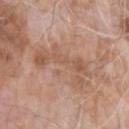Context:
A male subject approximately 75 years of age. Imaged with white-light lighting. Approximately 6.5 mm at its widest. Cropped from a total-body skin-imaging series; the visible field is about 15 mm. Automated tile analysis of the lesion measured a lesion–skin lightness drop of about 8 and a normalized lesion–skin contrast near 5.5. It also reported a classifier nevus-likeness of about 0/100 and a detector confidence of about 95 out of 100 that the crop contains a lesion. Located on the left forearm.